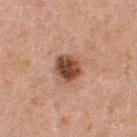notes: imaged on a skin check; not biopsied | lighting: white-light illumination | diameter: ≈3 mm | site: the front of the torso | subject: male, aged 53 to 57 | image source: total-body-photography crop, ~15 mm field of view.